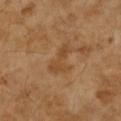Impression:
The lesion was tiled from a total-body skin photograph and was not biopsied.
Acquisition and patient details:
Approximately 4 mm at its widest. A close-up tile cropped from a whole-body skin photograph, about 15 mm across. Captured under cross-polarized illumination. The lesion is on the right forearm. The patient is a female aged 68–72.